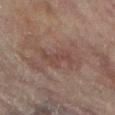| field | value |
|---|---|
| workup | total-body-photography surveillance lesion; no biopsy |
| anatomic site | the right leg |
| diameter | ~4 mm (longest diameter) |
| subject | female, aged approximately 80 |
| image | ~15 mm tile from a whole-body skin photo |
| lighting | cross-polarized |
| automated metrics | an area of roughly 4.5 mm² and a symmetry-axis asymmetry near 0.45; about 6 CIELAB-L* units darker than the surrounding skin; an automated nevus-likeness rating near 0 out of 100 and a lesion-detection confidence of about 95/100 |